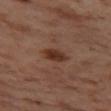{
  "biopsy_status": "not biopsied; imaged during a skin examination",
  "automated_metrics": {
    "vs_skin_darker_L": 10.0,
    "vs_skin_contrast_norm": 9.5,
    "nevus_likeness_0_100": 95
  },
  "patient": {
    "sex": "female",
    "age_approx": 55
  },
  "site": "left thigh",
  "lighting": "cross-polarized",
  "lesion_size": {
    "long_diameter_mm_approx": 3.0
  },
  "image": {
    "source": "total-body photography crop",
    "field_of_view_mm": 15
  }
}